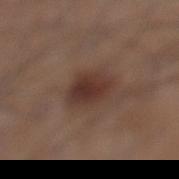Q: Was a biopsy performed?
A: catalogued during a skin exam; not biopsied
Q: Lesion location?
A: the left lower leg
Q: What is the imaging modality?
A: total-body-photography crop, ~15 mm field of view
Q: Patient demographics?
A: male, in their mid- to late 50s
Q: Automated lesion metrics?
A: a border-irregularity index near 2/10, a color-variation rating of about 3.5/10, and radial color variation of about 1; a classifier nevus-likeness of about 95/100 and lesion-presence confidence of about 100/100
Q: Illumination type?
A: white-light